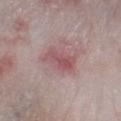Assessment: Recorded during total-body skin imaging; not selected for excision or biopsy. Context: This is a white-light tile. On the right lower leg. A 15 mm crop from a total-body photograph taken for skin-cancer surveillance. Approximately 3.5 mm at its widest. A male subject aged approximately 65.